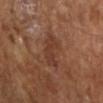biopsy_status: not biopsied; imaged during a skin examination
automated_metrics:
  area_mm2_approx: 6.5
  eccentricity: 0.85
  shape_asymmetry: 0.3
  vs_skin_darker_L: 6.0
  nevus_likeness_0_100: 0
  lesion_detection_confidence_0_100: 100
site: right forearm
patient:
  sex: female
  age_approx: 70
image:
  source: total-body photography crop
  field_of_view_mm: 15